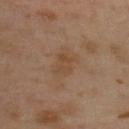The lesion is on the upper back. About 3 mm across. An algorithmic analysis of the crop reported an area of roughly 4.5 mm² and a shape-asymmetry score of about 0.35 (0 = symmetric). It also reported a border-irregularity index near 3.5/10, a color-variation rating of about 2/10, and peripheral color asymmetry of about 0.5. The software also gave a classifier nevus-likeness of about 0/100 and a lesion-detection confidence of about 100/100. A region of skin cropped from a whole-body photographic capture, roughly 15 mm wide. The tile uses cross-polarized illumination. The patient is a female aged 38–42.